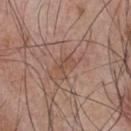Assessment: Captured during whole-body skin photography for melanoma surveillance; the lesion was not biopsied. Background: The patient is a male approximately 55 years of age. The lesion-visualizer software estimated a lesion area of about 4 mm² and a shape-asymmetry score of about 0.4 (0 = symmetric). And it measured a mean CIELAB color near L≈49 a*≈19 b*≈27, about 6 CIELAB-L* units darker than the surrounding skin, and a normalized lesion–skin contrast near 5. The tile uses white-light illumination. The lesion is located on the chest. A roughly 15 mm field-of-view crop from a total-body skin photograph. Measured at roughly 3 mm in maximum diameter.